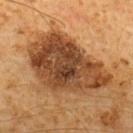follow-up: total-body-photography surveillance lesion; no biopsy
illumination: cross-polarized
lesion diameter: about 10.5 mm
site: the upper back
automated lesion analysis: an automated nevus-likeness rating near 0 out of 100
image source: ~15 mm tile from a whole-body skin photo
patient: male, approximately 60 years of age